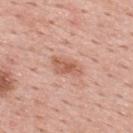Imaged during a routine full-body skin examination; the lesion was not biopsied and no histopathology is available. Cropped from a whole-body photographic skin survey; the tile spans about 15 mm. About 4 mm across. Located on the upper back. An algorithmic analysis of the crop reported a classifier nevus-likeness of about 50/100. A male subject aged approximately 55. Imaged with white-light lighting.